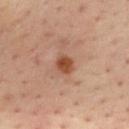Findings:
• biopsy status: total-body-photography surveillance lesion; no biopsy
• site: the mid back
• acquisition: total-body-photography crop, ~15 mm field of view
• subject: male, roughly 45 years of age